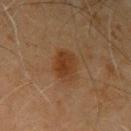Q: Was this lesion biopsied?
A: total-body-photography surveillance lesion; no biopsy
Q: What did automated image analysis measure?
A: an automated nevus-likeness rating near 85 out of 100 and a lesion-detection confidence of about 100/100
Q: What is the lesion's diameter?
A: ~4 mm (longest diameter)
Q: Where on the body is the lesion?
A: the left upper arm
Q: Patient demographics?
A: male, about 70 years old
Q: What kind of image is this?
A: 15 mm crop, total-body photography